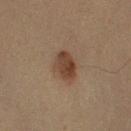subject: male, aged approximately 50
imaging modality: total-body-photography crop, ~15 mm field of view
lesion diameter: ~3.5 mm (longest diameter)
body site: the right upper arm
lighting: cross-polarized
automated lesion analysis: a border-irregularity index near 1.5/10, internal color variation of about 3 on a 0–10 scale, and peripheral color asymmetry of about 1